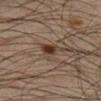| key | value |
|---|---|
| notes | imaged on a skin check; not biopsied |
| lesion diameter | ≈3.5 mm |
| subject | male, aged 33–37 |
| TBP lesion metrics | an average lesion color of about L≈34 a*≈14 b*≈23 (CIELAB) and a lesion-to-skin contrast of about 9 (normalized; higher = more distinct); a classifier nevus-likeness of about 95/100 |
| imaging modality | ~15 mm crop, total-body skin-cancer survey |
| site | the right lower leg |
| tile lighting | cross-polarized |